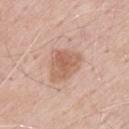The lesion was tiled from a total-body skin photograph and was not biopsied. A male subject aged approximately 70. Approximately 4.5 mm at its widest. This is a white-light tile. Cropped from a total-body skin-imaging series; the visible field is about 15 mm. On the upper back.